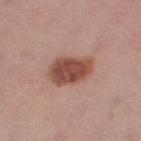Q: Is there a histopathology result?
A: catalogued during a skin exam; not biopsied